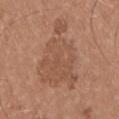<lesion>
<biopsy_status>not biopsied; imaged during a skin examination</biopsy_status>
<site>chest</site>
<patient>
  <sex>male</sex>
  <age_approx>25</age_approx>
</patient>
<image>
  <source>total-body photography crop</source>
  <field_of_view_mm>15</field_of_view_mm>
</image>
</lesion>